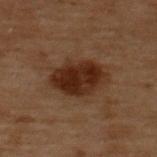  biopsy_status: not biopsied; imaged during a skin examination